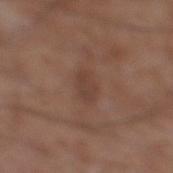{
  "biopsy_status": "not biopsied; imaged during a skin examination",
  "patient": {
    "sex": "male",
    "age_approx": 55
  },
  "lesion_size": {
    "long_diameter_mm_approx": 2.5
  },
  "site": "right lower leg",
  "lighting": "white-light",
  "automated_metrics": {
    "border_irregularity_0_10": 2.5,
    "color_variation_0_10": 2.0
  },
  "image": {
    "source": "total-body photography crop",
    "field_of_view_mm": 15
  }
}